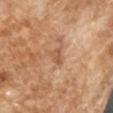  biopsy_status: not biopsied; imaged during a skin examination
  patient:
    sex: male
    age_approx: 65
  lighting: cross-polarized
  image:
    source: total-body photography crop
    field_of_view_mm: 15
  lesion_size:
    long_diameter_mm_approx: 2.5